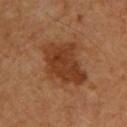Impression: No biopsy was performed on this lesion — it was imaged during a full skin examination and was not determined to be concerning. Image and clinical context: A male subject, about 65 years old. Cropped from a total-body skin-imaging series; the visible field is about 15 mm. From the upper back. Approximately 6 mm at its widest.